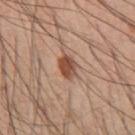| key | value |
|---|---|
| biopsy status | total-body-photography surveillance lesion; no biopsy |
| location | the abdomen |
| image-analysis metrics | an area of roughly 5 mm², an outline eccentricity of about 0.7 (0 = round, 1 = elongated), and a shape-asymmetry score of about 0.15 (0 = symmetric); a border-irregularity rating of about 1.5/10 and a within-lesion color-variation index near 4/10; a nevus-likeness score of about 95/100 |
| lighting | white-light |
| subject | male, aged 58–62 |
| diameter | ~3 mm (longest diameter) |
| imaging modality | ~15 mm crop, total-body skin-cancer survey |